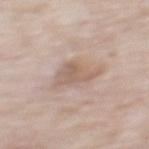Case summary:
• workup — total-body-photography surveillance lesion; no biopsy
• image source — total-body-photography crop, ~15 mm field of view
• body site — the mid back
• illumination — white-light
• diameter — ≈4 mm
• subject — male, aged 58–62